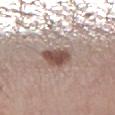Recorded during total-body skin imaging; not selected for excision or biopsy. Located on the leg. A 15 mm close-up tile from a total-body photography series done for melanoma screening. A male patient, in their 60s.